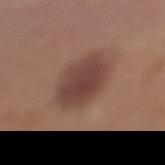<lesion>
  <biopsy_status>not biopsied; imaged during a skin examination</biopsy_status>
  <automated_metrics>
    <eccentricity>0.5</eccentricity>
    <shape_asymmetry>0.15</shape_asymmetry>
  </automated_metrics>
  <site>left lower leg</site>
  <patient>
    <sex>female</sex>
    <age_approx>55</age_approx>
  </patient>
  <lesion_size>
    <long_diameter_mm_approx>5.0</long_diameter_mm_approx>
  </lesion_size>
  <image>
    <source>total-body photography crop</source>
    <field_of_view_mm>15</field_of_view_mm>
  </image>
</lesion>